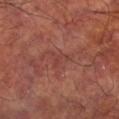This lesion was catalogued during total-body skin photography and was not selected for biopsy. The lesion-visualizer software estimated a lesion area of about 4 mm², a shape eccentricity near 0.7, and two-axis asymmetry of about 0.65. It also reported a mean CIELAB color near L≈40 a*≈26 b*≈25 and about 5 CIELAB-L* units darker than the surrounding skin. It also reported internal color variation of about 1.5 on a 0–10 scale and a peripheral color-asymmetry measure near 0.5. The analysis additionally found an automated nevus-likeness rating near 0 out of 100 and a lesion-detection confidence of about 75/100. The lesion's longest dimension is about 3 mm. From the leg. This is a cross-polarized tile. A region of skin cropped from a whole-body photographic capture, roughly 15 mm wide. The patient is a male aged 58–62.